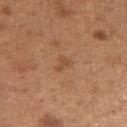workup: imaged on a skin check; not biopsied | imaging modality: 15 mm crop, total-body photography | size: ~2.5 mm (longest diameter) | patient: female, approximately 30 years of age | location: the left upper arm | automated metrics: an outline eccentricity of about 0.85 (0 = round, 1 = elongated) and a symmetry-axis asymmetry near 0.5; a classifier nevus-likeness of about 0/100 and a lesion-detection confidence of about 100/100.